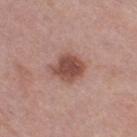Q: Was a biopsy performed?
A: imaged on a skin check; not biopsied
Q: Lesion location?
A: the right thigh
Q: What did automated image analysis measure?
A: a lesion area of about 9.5 mm² and an eccentricity of roughly 0.5; an average lesion color of about L≈49 a*≈22 b*≈25 (CIELAB), a lesion–skin lightness drop of about 13, and a lesion-to-skin contrast of about 9.5 (normalized; higher = more distinct)
Q: What is the imaging modality?
A: ~15 mm tile from a whole-body skin photo
Q: Patient demographics?
A: female, roughly 40 years of age